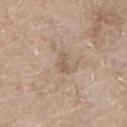follow-up: catalogued during a skin exam; not biopsied
location: the chest
acquisition: total-body-photography crop, ~15 mm field of view
illumination: white-light
lesion size: ≈2.5 mm
patient: male, approximately 65 years of age